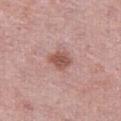Impression: This lesion was catalogued during total-body skin photography and was not selected for biopsy. Clinical summary: The tile uses white-light illumination. A female subject, aged approximately 65. A region of skin cropped from a whole-body photographic capture, roughly 15 mm wide. About 3 mm across. On the right thigh.